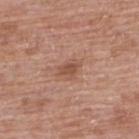biopsy status: catalogued during a skin exam; not biopsied | body site: the upper back | illumination: white-light | image: total-body-photography crop, ~15 mm field of view | subject: female, aged around 60 | diameter: about 3 mm.